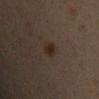{
  "lighting": "cross-polarized",
  "automated_metrics": {
    "border_irregularity_0_10": 2.5,
    "color_variation_0_10": 2.0,
    "peripheral_color_asymmetry": 0.5
  },
  "site": "chest",
  "image": {
    "source": "total-body photography crop",
    "field_of_view_mm": 15
  },
  "patient": {
    "sex": "male",
    "age_approx": 30
  },
  "lesion_size": {
    "long_diameter_mm_approx": 3.0
  }
}